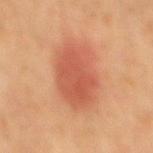- follow-up · total-body-photography surveillance lesion; no biopsy
- imaging modality · ~15 mm tile from a whole-body skin photo
- lesion size · ≈6 mm
- subject · male, about 65 years old
- illumination · cross-polarized
- location · the mid back